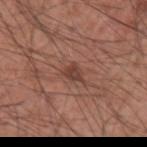Q: Was a biopsy performed?
A: total-body-photography surveillance lesion; no biopsy
Q: Who is the patient?
A: male, aged approximately 60
Q: Lesion size?
A: about 3 mm
Q: What is the anatomic site?
A: the arm
Q: Illumination type?
A: white-light illumination
Q: How was this image acquired?
A: ~15 mm tile from a whole-body skin photo